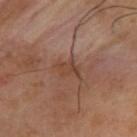Clinical impression: Recorded during total-body skin imaging; not selected for excision or biopsy. Acquisition and patient details: A lesion tile, about 15 mm wide, cut from a 3D total-body photograph. The lesion is on the upper back. A male patient aged 38 to 42.